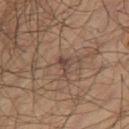Imaged during a routine full-body skin examination; the lesion was not biopsied and no histopathology is available.
Imaged with cross-polarized lighting.
On the right thigh.
A roughly 15 mm field-of-view crop from a total-body skin photograph.
Automated image analysis of the tile measured an area of roughly 3 mm², an eccentricity of roughly 0.65, and two-axis asymmetry of about 0.55. The software also gave an average lesion color of about L≈40 a*≈15 b*≈22 (CIELAB), about 7 CIELAB-L* units darker than the surrounding skin, and a normalized lesion–skin contrast near 7. And it measured a border-irregularity rating of about 5/10, internal color variation of about 2.5 on a 0–10 scale, and radial color variation of about 1. The software also gave a nevus-likeness score of about 0/100 and lesion-presence confidence of about 85/100.
The recorded lesion diameter is about 2.5 mm.
A male patient, about 65 years old.